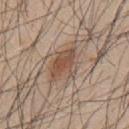No biopsy was performed on this lesion — it was imaged during a full skin examination and was not determined to be concerning. Cropped from a whole-body photographic skin survey; the tile spans about 15 mm. Longest diameter approximately 4.5 mm. A male patient, in their mid-40s. From the upper back. Imaged with white-light lighting.